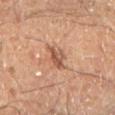• notes: catalogued during a skin exam; not biopsied
• body site: the left lower leg
• size: ~3 mm (longest diameter)
• patient: male, aged 58–62
• acquisition: ~15 mm tile from a whole-body skin photo
• illumination: cross-polarized
• image-analysis metrics: a footprint of about 5 mm², an eccentricity of roughly 0.7, and two-axis asymmetry of about 0.3; a lesion color around L≈49 a*≈21 b*≈28 in CIELAB and a normalized lesion–skin contrast near 7.5; a nevus-likeness score of about 25/100